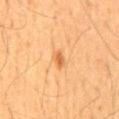The lesion was photographed on a routine skin check and not biopsied; there is no pathology result.
A male subject in their 60s.
The tile uses cross-polarized illumination.
Located on the back.
The total-body-photography lesion software estimated border irregularity of about 3 on a 0–10 scale and internal color variation of about 0.5 on a 0–10 scale.
A 15 mm close-up tile from a total-body photography series done for melanoma screening.
Measured at roughly 2 mm in maximum diameter.